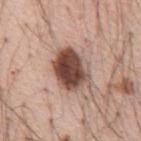Findings:
* biopsy status · imaged on a skin check; not biopsied
* location · the front of the torso
* size · ~5.5 mm (longest diameter)
* patient · male, approximately 55 years of age
* illumination · white-light
* automated metrics · a lesion color around L≈47 a*≈20 b*≈24 in CIELAB, a lesion–skin lightness drop of about 20, and a normalized lesion–skin contrast near 13.5
* imaging modality · ~15 mm tile from a whole-body skin photo